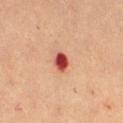Case summary:
* follow-up — catalogued during a skin exam; not biopsied
* illumination — cross-polarized illumination
* size — about 2.5 mm
* body site — the right thigh
* subject — female, aged 68–72
* image — ~15 mm tile from a whole-body skin photo
* automated lesion analysis — a lesion-detection confidence of about 100/100